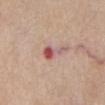The lesion was tiled from a total-body skin photograph and was not biopsied.
A close-up tile cropped from a whole-body skin photograph, about 15 mm across.
Imaged with white-light lighting.
About 3.5 mm across.
A male patient, aged 78 to 82.
On the front of the torso.